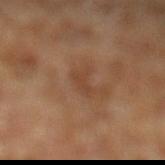{"biopsy_status": "not biopsied; imaged during a skin examination", "automated_metrics": {"area_mm2_approx": 2.5, "eccentricity": 0.95, "shape_asymmetry": 0.5, "cielab_L": 41, "cielab_a": 20, "cielab_b": 30, "vs_skin_darker_L": 6.0, "vs_skin_contrast_norm": 5.0, "nevus_likeness_0_100": 0}, "image": {"source": "total-body photography crop", "field_of_view_mm": 15}, "lesion_size": {"long_diameter_mm_approx": 3.0}, "patient": {"sex": "male", "age_approx": 70}, "site": "left lower leg", "lighting": "cross-polarized"}